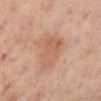Clinical impression:
Recorded during total-body skin imaging; not selected for excision or biopsy.
Clinical summary:
The patient is a female in their 40s. From the right lower leg. A lesion tile, about 15 mm wide, cut from a 3D total-body photograph.